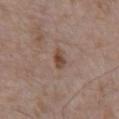Case summary:
• notes — total-body-photography surveillance lesion; no biopsy
• automated metrics — a lesion area of about 3.5 mm², an eccentricity of roughly 0.75, and two-axis asymmetry of about 0.25; border irregularity of about 2 on a 0–10 scale, a within-lesion color-variation index near 2.5/10, and radial color variation of about 0.5; a lesion-detection confidence of about 100/100
• patient — male, about 70 years old
• tile lighting — white-light
• site — the abdomen
• acquisition — 15 mm crop, total-body photography
• lesion diameter — ~2.5 mm (longest diameter)